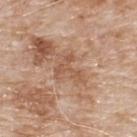follow-up: no biopsy performed (imaged during a skin exam); lesion diameter: ≈3.5 mm; site: the upper back; tile lighting: white-light illumination; image: 15 mm crop, total-body photography; patient: male, approximately 80 years of age.